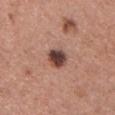Clinical impression:
The lesion was photographed on a routine skin check and not biopsied; there is no pathology result.
Acquisition and patient details:
About 3 mm across. Cropped from a whole-body photographic skin survey; the tile spans about 15 mm. The lesion is located on the front of the torso. The total-body-photography lesion software estimated an area of roughly 6 mm² and a shape eccentricity near 0.4. It also reported a border-irregularity rating of about 2.5/10 and radial color variation of about 2.5. The analysis additionally found a nevus-likeness score of about 60/100. A male patient, aged approximately 45. Imaged with white-light lighting.